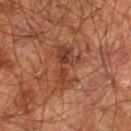Notes:
* image source · ~15 mm tile from a whole-body skin photo
* diameter · ~5.5 mm (longest diameter)
* automated metrics · an area of roughly 10 mm², an eccentricity of roughly 0.9, and two-axis asymmetry of about 0.45; a mean CIELAB color near L≈39 a*≈24 b*≈31, about 9 CIELAB-L* units darker than the surrounding skin, and a normalized lesion–skin contrast near 7; a border-irregularity rating of about 7.5/10; a classifier nevus-likeness of about 0/100 and a detector confidence of about 95 out of 100 that the crop contains a lesion
* patient · male, roughly 60 years of age
* body site · the right leg
* tile lighting · cross-polarized